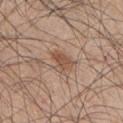Assessment:
The lesion was tiled from a total-body skin photograph and was not biopsied.
Clinical summary:
The patient is a male in their mid-40s. Located on the upper back. A close-up tile cropped from a whole-body skin photograph, about 15 mm across. Automated tile analysis of the lesion measured an area of roughly 4.5 mm², a shape eccentricity near 0.7, and a symmetry-axis asymmetry near 0.45. The analysis additionally found an automated nevus-likeness rating near 65 out of 100 and a detector confidence of about 100 out of 100 that the crop contains a lesion. The tile uses white-light illumination. Approximately 3 mm at its widest.